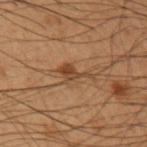Recorded during total-body skin imaging; not selected for excision or biopsy. The subject is a male roughly 55 years of age. A region of skin cropped from a whole-body photographic capture, roughly 15 mm wide. Automated tile analysis of the lesion measured a lesion area of about 4.5 mm² and a symmetry-axis asymmetry near 0.6. And it measured a nevus-likeness score of about 5/100 and a detector confidence of about 100 out of 100 that the crop contains a lesion. The lesion is on the left upper arm.